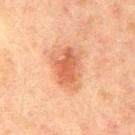Impression:
Recorded during total-body skin imaging; not selected for excision or biopsy.
Background:
A 15 mm close-up tile from a total-body photography series done for melanoma screening. Located on the right upper arm. The patient is a male approximately 45 years of age.